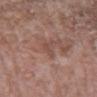Recorded during total-body skin imaging; not selected for excision or biopsy. An algorithmic analysis of the crop reported a footprint of about 3 mm², a shape eccentricity near 0.9, and a shape-asymmetry score of about 0.45 (0 = symmetric). Approximately 2.5 mm at its widest. A female subject, aged approximately 70. The tile uses white-light illumination. On the arm. A 15 mm close-up tile from a total-body photography series done for melanoma screening.